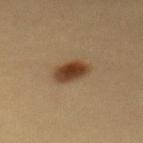• workup · total-body-photography surveillance lesion; no biopsy
• image source · 15 mm crop, total-body photography
• site · the back
• lighting · cross-polarized illumination
• patient · female, roughly 30 years of age